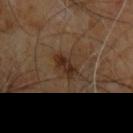This lesion was catalogued during total-body skin photography and was not selected for biopsy.
Longest diameter approximately 3.5 mm.
A region of skin cropped from a whole-body photographic capture, roughly 15 mm wide.
The total-body-photography lesion software estimated an area of roughly 6.5 mm², an outline eccentricity of about 0.6 (0 = round, 1 = elongated), and a symmetry-axis asymmetry near 0.15. And it measured a border-irregularity index near 2/10, internal color variation of about 3.5 on a 0–10 scale, and radial color variation of about 1. It also reported a nevus-likeness score of about 80/100.
A male patient, approximately 60 years of age.
From the chest.
The tile uses cross-polarized illumination.